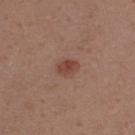Q: Is there a histopathology result?
A: catalogued during a skin exam; not biopsied
Q: What is the lesion's diameter?
A: ~2.5 mm (longest diameter)
Q: What is the anatomic site?
A: the leg
Q: Who is the patient?
A: female, approximately 30 years of age
Q: How was this image acquired?
A: 15 mm crop, total-body photography
Q: What did automated image analysis measure?
A: a lesion color around L≈44 a*≈22 b*≈26 in CIELAB; a border-irregularity rating of about 1.5/10, a within-lesion color-variation index near 2/10, and peripheral color asymmetry of about 0.5; an automated nevus-likeness rating near 90 out of 100 and a lesion-detection confidence of about 100/100
Q: What lighting was used for the tile?
A: white-light illumination